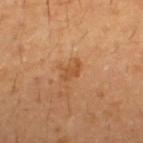Part of a total-body skin-imaging series; this lesion was reviewed on a skin check and was not flagged for biopsy.
The subject is a male aged approximately 30.
Automated image analysis of the tile measured a footprint of about 3.5 mm², an outline eccentricity of about 0.85 (0 = round, 1 = elongated), and a symmetry-axis asymmetry near 0.3. And it measured a lesion color around L≈50 a*≈23 b*≈39 in CIELAB, a lesion–skin lightness drop of about 8, and a normalized border contrast of about 6. The software also gave a border-irregularity index near 3.5/10, internal color variation of about 0.5 on a 0–10 scale, and a peripheral color-asymmetry measure near 0.
A roughly 15 mm field-of-view crop from a total-body skin photograph.
On the upper back.
Approximately 3 mm at its widest.